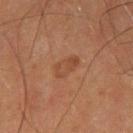Impression: The lesion was photographed on a routine skin check and not biopsied; there is no pathology result. Background: A 15 mm close-up extracted from a 3D total-body photography capture. The tile uses cross-polarized illumination. A male patient in their 60s. Located on the right thigh. Longest diameter approximately 3.5 mm.